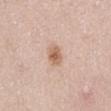Clinical impression:
The lesion was photographed on a routine skin check and not biopsied; there is no pathology result.